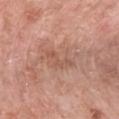{"biopsy_status": "not biopsied; imaged during a skin examination", "lesion_size": {"long_diameter_mm_approx": 4.0}, "automated_metrics": {"border_irregularity_0_10": 8.0, "lesion_detection_confidence_0_100": 100}, "image": {"source": "total-body photography crop", "field_of_view_mm": 15}, "site": "chest", "lighting": "white-light", "patient": {"sex": "female", "age_approx": 40}}